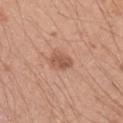Assessment:
This lesion was catalogued during total-body skin photography and was not selected for biopsy.
Image and clinical context:
The total-body-photography lesion software estimated two-axis asymmetry of about 0.2. The software also gave an average lesion color of about L≈55 a*≈23 b*≈31 (CIELAB), a lesion–skin lightness drop of about 10, and a lesion-to-skin contrast of about 7 (normalized; higher = more distinct). The analysis additionally found border irregularity of about 2 on a 0–10 scale, a color-variation rating of about 2.5/10, and peripheral color asymmetry of about 1. On the right upper arm. About 3 mm across. A 15 mm crop from a total-body photograph taken for skin-cancer surveillance. Captured under white-light illumination. A male subject in their 20s.